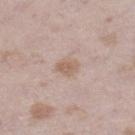Case summary:
• notes — imaged on a skin check; not biopsied